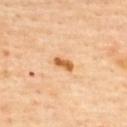The lesion was tiled from a total-body skin photograph and was not biopsied.
A close-up tile cropped from a whole-body skin photograph, about 15 mm across.
The tile uses cross-polarized illumination.
The lesion's longest dimension is about 2.5 mm.
From the upper back.
A female patient, aged 58–62.
An algorithmic analysis of the crop reported an area of roughly 3.5 mm², an eccentricity of roughly 0.85, and a shape-asymmetry score of about 0.25 (0 = symmetric).